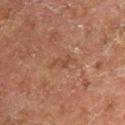| key | value |
|---|---|
| follow-up | total-body-photography surveillance lesion; no biopsy |
| lighting | cross-polarized |
| acquisition | total-body-photography crop, ~15 mm field of view |
| body site | the right lower leg |
| automated lesion analysis | a border-irregularity index near 5.5/10 and a peripheral color-asymmetry measure near 0; a classifier nevus-likeness of about 0/100 and a detector confidence of about 100 out of 100 that the crop contains a lesion |
| subject | male, roughly 80 years of age |